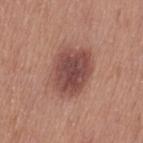Part of a total-body skin-imaging series; this lesion was reviewed on a skin check and was not flagged for biopsy.
A female subject about 40 years old.
A lesion tile, about 15 mm wide, cut from a 3D total-body photograph.
From the left thigh.
The recorded lesion diameter is about 5 mm.
Imaged with white-light lighting.